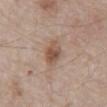  biopsy_status: not biopsied; imaged during a skin examination
  lighting: white-light
  image:
    source: total-body photography crop
    field_of_view_mm: 15
  site: abdomen
  lesion_size:
    long_diameter_mm_approx: 3.5
  patient:
    sex: male
    age_approx: 80
  automated_metrics:
    area_mm2_approx: 6.0
    eccentricity: 0.7
    shape_asymmetry: 0.25
    cielab_L: 52
    cielab_a: 18
    cielab_b: 28
    vs_skin_contrast_norm: 7.5
    nevus_likeness_0_100: 60
    lesion_detection_confidence_0_100: 100